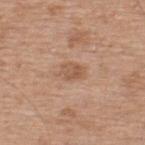This lesion was catalogued during total-body skin photography and was not selected for biopsy. This is a white-light tile. Automated image analysis of the tile measured a mean CIELAB color near L≈56 a*≈20 b*≈32 and a lesion–skin lightness drop of about 8. The subject is a male aged around 55. A 15 mm close-up extracted from a 3D total-body photography capture. On the upper back. The recorded lesion diameter is about 3 mm.